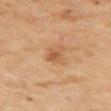Imaged during a routine full-body skin examination; the lesion was not biopsied and no histopathology is available.
Cropped from a whole-body photographic skin survey; the tile spans about 15 mm.
Located on the right upper arm.
A male patient, roughly 45 years of age.
Measured at roughly 3.5 mm in maximum diameter.